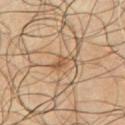Assessment:
The lesion was tiled from a total-body skin photograph and was not biopsied.
Clinical summary:
A male subject, aged 63–67. Measured at roughly 3 mm in maximum diameter. On the chest. Imaged with cross-polarized lighting. A 15 mm crop from a total-body photograph taken for skin-cancer surveillance. The total-body-photography lesion software estimated an area of roughly 3.5 mm², an eccentricity of roughly 0.85, and a shape-asymmetry score of about 0.4 (0 = symmetric). The software also gave a lesion color around L≈52 a*≈17 b*≈33 in CIELAB, roughly 8 lightness units darker than nearby skin, and a normalized border contrast of about 6.5.